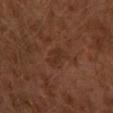Imaged during a routine full-body skin examination; the lesion was not biopsied and no histopathology is available.
A male patient, about 30 years old.
A 15 mm crop from a total-body photograph taken for skin-cancer surveillance.
About 2.5 mm across.
The lesion is on the left lower leg.